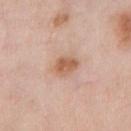biopsy status: imaged on a skin check; not biopsied
diameter: ~3 mm (longest diameter)
lighting: white-light
subject: male, roughly 55 years of age
anatomic site: the chest
image: ~15 mm tile from a whole-body skin photo